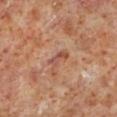The total-body-photography lesion software estimated a lesion color around L≈47 a*≈23 b*≈27 in CIELAB, a lesion–skin lightness drop of about 8, and a normalized border contrast of about 6.5. It also reported a border-irregularity rating of about 5/10 and a within-lesion color-variation index near 0/10. The analysis additionally found a nevus-likeness score of about 0/100 and a detector confidence of about 100 out of 100 that the crop contains a lesion. Cropped from a total-body skin-imaging series; the visible field is about 15 mm. The patient is a male aged approximately 60. The lesion is located on the left lower leg. The recorded lesion diameter is about 3 mm.